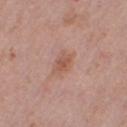This lesion was catalogued during total-body skin photography and was not selected for biopsy. The patient is a female aged 38–42. The total-body-photography lesion software estimated a lesion area of about 5 mm², an outline eccentricity of about 0.7 (0 = round, 1 = elongated), and a shape-asymmetry score of about 0.2 (0 = symmetric). The software also gave a mean CIELAB color near L≈55 a*≈22 b*≈28, roughly 8 lightness units darker than nearby skin, and a normalized border contrast of about 6. And it measured a border-irregularity index near 2.5/10 and a color-variation rating of about 3/10. This is a white-light tile. Cropped from a whole-body photographic skin survey; the tile spans about 15 mm. On the right thigh. The lesion's longest dimension is about 3.5 mm.